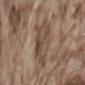<lesion>
<biopsy_status>not biopsied; imaged during a skin examination</biopsy_status>
<lighting>white-light</lighting>
<image>
  <source>total-body photography crop</source>
  <field_of_view_mm>15</field_of_view_mm>
</image>
<lesion_size>
  <long_diameter_mm_approx>5.0</long_diameter_mm_approx>
</lesion_size>
<patient>
  <sex>male</sex>
  <age_approx>75</age_approx>
</patient>
<site>back</site>
</lesion>